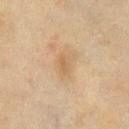workup: imaged on a skin check; not biopsied
anatomic site: the chest
patient: male, in their 70s
TBP lesion metrics: a lesion area of about 3 mm² and a symmetry-axis asymmetry near 0.15; about 6 CIELAB-L* units darker than the surrounding skin and a normalized lesion–skin contrast near 5
lighting: cross-polarized illumination
lesion size: about 2.5 mm
image: total-body-photography crop, ~15 mm field of view